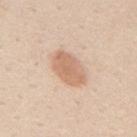Clinical impression:
Imaged during a routine full-body skin examination; the lesion was not biopsied and no histopathology is available.
Background:
Measured at roughly 4.5 mm in maximum diameter. A female subject about 45 years old. The lesion is located on the mid back. The lesion-visualizer software estimated an area of roughly 10 mm² and a shape eccentricity near 0.8. And it measured a lesion color around L≈66 a*≈19 b*≈32 in CIELAB and a lesion-to-skin contrast of about 7 (normalized; higher = more distinct). It also reported a classifier nevus-likeness of about 75/100. A close-up tile cropped from a whole-body skin photograph, about 15 mm across. Imaged with white-light lighting.